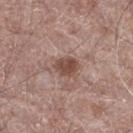Part of a total-body skin-imaging series; this lesion was reviewed on a skin check and was not flagged for biopsy. The patient is a male aged 68 to 72. A 15 mm close-up tile from a total-body photography series done for melanoma screening. On the right thigh.